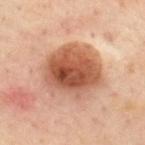The lesion was tiled from a total-body skin photograph and was not biopsied.
From the upper back.
A roughly 15 mm field-of-view crop from a total-body skin photograph.
A male subject aged around 45.
The recorded lesion diameter is about 7 mm.